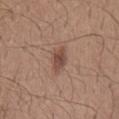Recorded during total-body skin imaging; not selected for excision or biopsy.
Captured under white-light illumination.
About 3.5 mm across.
A male patient aged approximately 25.
The lesion-visualizer software estimated a border-irregularity index near 2.5/10, internal color variation of about 3 on a 0–10 scale, and peripheral color asymmetry of about 1. The analysis additionally found a classifier nevus-likeness of about 85/100.
A roughly 15 mm field-of-view crop from a total-body skin photograph.
Located on the mid back.